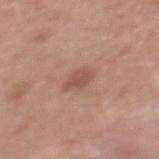The lesion was tiled from a total-body skin photograph and was not biopsied.
Captured under white-light illumination.
A region of skin cropped from a whole-body photographic capture, roughly 15 mm wide.
The subject is a female aged around 35.
Located on the upper back.